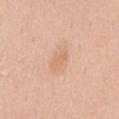Captured during whole-body skin photography for melanoma surveillance; the lesion was not biopsied. Automated image analysis of the tile measured an area of roughly 4.5 mm² and a shape eccentricity near 0.8. The lesion's longest dimension is about 3 mm. Imaged with white-light lighting. A female patient, aged 48 to 52. A 15 mm crop from a total-body photograph taken for skin-cancer surveillance. On the mid back.